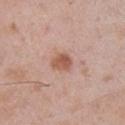Captured during whole-body skin photography for melanoma surveillance; the lesion was not biopsied. This image is a 15 mm lesion crop taken from a total-body photograph. Automated tile analysis of the lesion measured a mean CIELAB color near L≈56 a*≈22 b*≈29 and about 11 CIELAB-L* units darker than the surrounding skin. The lesion is located on the right upper arm. The tile uses white-light illumination. The subject is a male aged approximately 55.